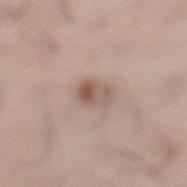{
  "biopsy_status": "not biopsied; imaged during a skin examination",
  "patient": {
    "sex": "male",
    "age_approx": 35
  },
  "image": {
    "source": "total-body photography crop",
    "field_of_view_mm": 15
  },
  "site": "left lower leg",
  "lighting": "white-light",
  "lesion_size": {
    "long_diameter_mm_approx": 3.5
  }
}